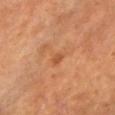The lesion was tiled from a total-body skin photograph and was not biopsied.
Captured under cross-polarized illumination.
From the head or neck.
Cropped from a total-body skin-imaging series; the visible field is about 15 mm.
The total-body-photography lesion software estimated an average lesion color of about L≈54 a*≈25 b*≈38 (CIELAB), about 6 CIELAB-L* units darker than the surrounding skin, and a normalized border contrast of about 4.5. The analysis additionally found a border-irregularity rating of about 4/10, internal color variation of about 1 on a 0–10 scale, and a peripheral color-asymmetry measure near 0.5.
Measured at roughly 2.5 mm in maximum diameter.
A female patient aged approximately 65.